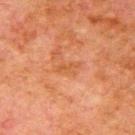Assessment:
No biopsy was performed on this lesion — it was imaged during a full skin examination and was not determined to be concerning.
Context:
Approximately 2.5 mm at its widest. A male patient aged 78 to 82. A 15 mm close-up extracted from a 3D total-body photography capture. This is a cross-polarized tile. The total-body-photography lesion software estimated a footprint of about 2.5 mm², an eccentricity of roughly 0.9, and a shape-asymmetry score of about 0.3 (0 = symmetric). It also reported an average lesion color of about L≈44 a*≈23 b*≈34 (CIELAB), a lesion–skin lightness drop of about 5, and a normalized lesion–skin contrast near 5. The lesion is located on the right upper arm.